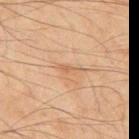Q: Was a biopsy performed?
A: no biopsy performed (imaged during a skin exam)
Q: What lighting was used for the tile?
A: cross-polarized
Q: What is the lesion's diameter?
A: about 3 mm
Q: What kind of image is this?
A: total-body-photography crop, ~15 mm field of view
Q: What are the patient's age and sex?
A: male, aged approximately 45
Q: Where on the body is the lesion?
A: the mid back